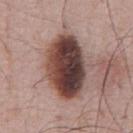| key | value |
|---|---|
| biopsy status | imaged on a skin check; not biopsied |
| patient | male, aged 53–57 |
| image source | 15 mm crop, total-body photography |